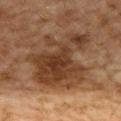follow-up — imaged on a skin check; not biopsied | tile lighting — cross-polarized illumination | patient — female, in their 60s | body site — the upper back | TBP lesion metrics — a footprint of about 37 mm², an outline eccentricity of about 0.4 (0 = round, 1 = elongated), and a shape-asymmetry score of about 0.4 (0 = symmetric); a mean CIELAB color near L≈34 a*≈17 b*≈28, roughly 10 lightness units darker than nearby skin, and a normalized lesion–skin contrast near 9; internal color variation of about 5.5 on a 0–10 scale and peripheral color asymmetry of about 2; a nevus-likeness score of about 45/100 and lesion-presence confidence of about 100/100 | lesion diameter — ~8 mm (longest diameter) | acquisition — total-body-photography crop, ~15 mm field of view.